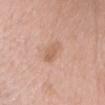The lesion was tiled from a total-body skin photograph and was not biopsied. Located on the head or neck. The subject is a female aged approximately 40. This is a white-light tile. A region of skin cropped from a whole-body photographic capture, roughly 15 mm wide. Measured at roughly 3.5 mm in maximum diameter. The total-body-photography lesion software estimated a color-variation rating of about 2/10 and a peripheral color-asymmetry measure near 0.5. And it measured lesion-presence confidence of about 100/100.